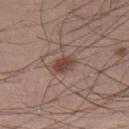Captured during whole-body skin photography for melanoma surveillance; the lesion was not biopsied. Automated image analysis of the tile measured a footprint of about 4 mm², an outline eccentricity of about 0.75 (0 = round, 1 = elongated), and a shape-asymmetry score of about 0.2 (0 = symmetric). And it measured border irregularity of about 2 on a 0–10 scale, internal color variation of about 3 on a 0–10 scale, and a peripheral color-asymmetry measure near 1. The software also gave a nevus-likeness score of about 90/100 and a lesion-detection confidence of about 100/100. Located on the leg. A close-up tile cropped from a whole-body skin photograph, about 15 mm across. Captured under white-light illumination. The subject is a male approximately 35 years of age.